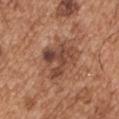Case summary:
• workup — catalogued during a skin exam; not biopsied
• tile lighting — white-light
• lesion diameter — ~5.5 mm (longest diameter)
• acquisition — ~15 mm tile from a whole-body skin photo
• body site — the right upper arm
• patient — male, roughly 55 years of age